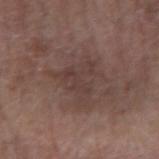Notes:
– anatomic site — the left forearm
– acquisition — total-body-photography crop, ~15 mm field of view
– lesion diameter — ~5.5 mm (longest diameter)
– subject — male, approximately 70 years of age
– tile lighting — white-light